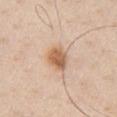No biopsy was performed on this lesion — it was imaged during a full skin examination and was not determined to be concerning. Imaged with white-light lighting. The lesion-visualizer software estimated a shape eccentricity near 0.55 and a symmetry-axis asymmetry near 0.15. It also reported about 12 CIELAB-L* units darker than the surrounding skin and a lesion-to-skin contrast of about 8 (normalized; higher = more distinct). And it measured a detector confidence of about 100 out of 100 that the crop contains a lesion. The patient is a male aged around 60. The lesion is on the chest. A 15 mm crop from a total-body photograph taken for skin-cancer surveillance. The recorded lesion diameter is about 3.5 mm.